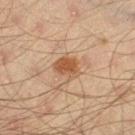| key | value |
|---|---|
| follow-up | no biopsy performed (imaged during a skin exam) |
| site | the left thigh |
| acquisition | ~15 mm crop, total-body skin-cancer survey |
| patient | male, approximately 45 years of age |
| lesion size | about 3 mm |
| tile lighting | cross-polarized illumination |